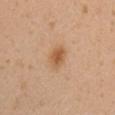patient — female, aged approximately 30 | lighting — white-light | TBP lesion metrics — peripheral color asymmetry of about 0.5; a classifier nevus-likeness of about 95/100 and lesion-presence confidence of about 100/100 | image source — total-body-photography crop, ~15 mm field of view | lesion diameter — about 3 mm | anatomic site — the right upper arm.